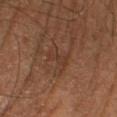Q: Is there a histopathology result?
A: total-body-photography surveillance lesion; no biopsy
Q: Patient demographics?
A: male, aged 63 to 67
Q: How was this image acquired?
A: ~15 mm crop, total-body skin-cancer survey
Q: What lighting was used for the tile?
A: cross-polarized illumination
Q: What is the anatomic site?
A: the left leg
Q: What did automated image analysis measure?
A: a lesion area of about 3 mm² and a shape eccentricity near 0.9; border irregularity of about 5 on a 0–10 scale, a color-variation rating of about 0/10, and peripheral color asymmetry of about 0; an automated nevus-likeness rating near 0 out of 100 and a lesion-detection confidence of about 95/100